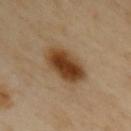| feature | finding |
|---|---|
| biopsy status | imaged on a skin check; not biopsied |
| imaging modality | total-body-photography crop, ~15 mm field of view |
| location | the upper back |
| tile lighting | cross-polarized |
| patient | female, about 60 years old |
| size | ≈5.5 mm |